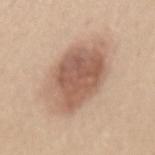follow-up: no biopsy performed (imaged during a skin exam) | body site: the mid back | lesion size: ≈8.5 mm | tile lighting: white-light illumination | patient: female, aged approximately 35 | imaging modality: ~15 mm crop, total-body skin-cancer survey.